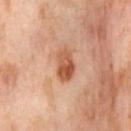A region of skin cropped from a whole-body photographic capture, roughly 15 mm wide.
A female patient roughly 55 years of age.
Automated image analysis of the tile measured a footprint of about 6.5 mm², an eccentricity of roughly 0.85, and a symmetry-axis asymmetry near 0.3. The software also gave an automated nevus-likeness rating near 50 out of 100 and a detector confidence of about 100 out of 100 that the crop contains a lesion.
Located on the right thigh.